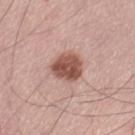Captured during whole-body skin photography for melanoma surveillance; the lesion was not biopsied.
A 15 mm close-up extracted from a 3D total-body photography capture.
On the left thigh.
The patient is a male approximately 70 years of age.